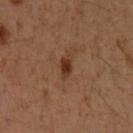{"biopsy_status": "not biopsied; imaged during a skin examination", "image": {"source": "total-body photography crop", "field_of_view_mm": 15}, "site": "left forearm", "patient": {"sex": "female", "age_approx": 55}}